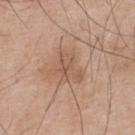Clinical impression: This lesion was catalogued during total-body skin photography and was not selected for biopsy. Background: About 4 mm across. A male patient approximately 55 years of age. Cropped from a total-body skin-imaging series; the visible field is about 15 mm. Imaged with white-light lighting. The lesion is located on the upper back. Automated tile analysis of the lesion measured a shape eccentricity near 0.7 and two-axis asymmetry of about 0.6. It also reported a mean CIELAB color near L≈56 a*≈19 b*≈30.